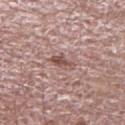The lesion was photographed on a routine skin check and not biopsied; there is no pathology result. The patient is a male in their 70s. The lesion is on the arm. A lesion tile, about 15 mm wide, cut from a 3D total-body photograph. Longest diameter approximately 3 mm. Imaged with white-light lighting. The lesion-visualizer software estimated a lesion area of about 4 mm², a shape eccentricity near 0.85, and a shape-asymmetry score of about 0.35 (0 = symmetric). And it measured a within-lesion color-variation index near 2.5/10 and a peripheral color-asymmetry measure near 1. And it measured an automated nevus-likeness rating near 0 out of 100.